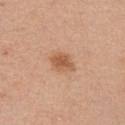Part of a total-body skin-imaging series; this lesion was reviewed on a skin check and was not flagged for biopsy.
Measured at roughly 2.5 mm in maximum diameter.
Located on the right upper arm.
This is a white-light tile.
This image is a 15 mm lesion crop taken from a total-body photograph.
The patient is a male aged around 30.